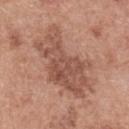Notes:
– workup — catalogued during a skin exam; not biopsied
– diameter — ~9 mm (longest diameter)
– subject — female, in their 60s
– body site — the back
– image source — ~15 mm tile from a whole-body skin photo